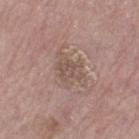Clinical impression:
Part of a total-body skin-imaging series; this lesion was reviewed on a skin check and was not flagged for biopsy.